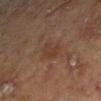The lesion was photographed on a routine skin check and not biopsied; there is no pathology result.
The total-body-photography lesion software estimated a footprint of about 4 mm², a shape eccentricity near 0.8, and a symmetry-axis asymmetry near 0.4. The software also gave a mean CIELAB color near L≈31 a*≈16 b*≈25, a lesion–skin lightness drop of about 5, and a normalized lesion–skin contrast near 5.5. The software also gave a border-irregularity rating of about 4/10 and a peripheral color-asymmetry measure near 0.5.
A roughly 15 mm field-of-view crop from a total-body skin photograph.
A female patient roughly 80 years of age.
Located on the left forearm.